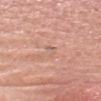biopsy status — imaged on a skin check; not biopsied
illumination — white-light illumination
imaging modality — ~15 mm crop, total-body skin-cancer survey
TBP lesion metrics — a lesion color around L≈62 a*≈20 b*≈26 in CIELAB, about 6 CIELAB-L* units darker than the surrounding skin, and a lesion-to-skin contrast of about 4 (normalized; higher = more distinct); an automated nevus-likeness rating near 0 out of 100 and a detector confidence of about 90 out of 100 that the crop contains a lesion
subject — male, about 55 years old
diameter — ~2 mm (longest diameter)
body site — the head or neck